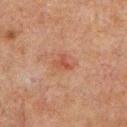biopsy status=imaged on a skin check; not biopsied | patient=male, aged around 60 | diameter=~2.5 mm (longest diameter) | automated lesion analysis=a lesion area of about 4 mm², a shape eccentricity near 0.7, and two-axis asymmetry of about 0.35; a border-irregularity rating of about 4/10, a color-variation rating of about 2.5/10, and a peripheral color-asymmetry measure near 1; a classifier nevus-likeness of about 10/100 and lesion-presence confidence of about 100/100 | body site=the chest | image=total-body-photography crop, ~15 mm field of view.